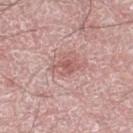Case summary:
* follow-up — catalogued during a skin exam; not biopsied
* automated lesion analysis — a lesion color around L≈57 a*≈24 b*≈23 in CIELAB, roughly 9 lightness units darker than nearby skin, and a normalized border contrast of about 6; an automated nevus-likeness rating near 5 out of 100 and a detector confidence of about 100 out of 100 that the crop contains a lesion
* tile lighting — white-light illumination
* location — the right thigh
* acquisition — 15 mm crop, total-body photography
* lesion size — ≈3 mm
* patient — male, roughly 50 years of age